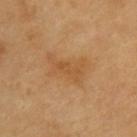Impression: This lesion was catalogued during total-body skin photography and was not selected for biopsy.